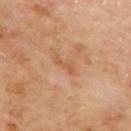Assessment: Recorded during total-body skin imaging; not selected for excision or biopsy. Image and clinical context: The patient is a male roughly 70 years of age. A close-up tile cropped from a whole-body skin photograph, about 15 mm across. Located on the mid back. This is a cross-polarized tile.